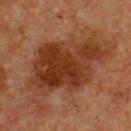Clinical impression: No biopsy was performed on this lesion — it was imaged during a full skin examination and was not determined to be concerning. Acquisition and patient details: Located on the front of the torso. This is a cross-polarized tile. The total-body-photography lesion software estimated an outline eccentricity of about 0.8 (0 = round, 1 = elongated) and a shape-asymmetry score of about 0.25 (0 = symmetric). And it measured an average lesion color of about L≈29 a*≈20 b*≈27 (CIELAB) and a lesion-to-skin contrast of about 9 (normalized; higher = more distinct). The analysis additionally found a classifier nevus-likeness of about 50/100. The patient is a male aged 73–77. A close-up tile cropped from a whole-body skin photograph, about 15 mm across.